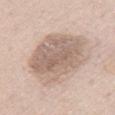follow-up=total-body-photography surveillance lesion; no biopsy
tile lighting=white-light
acquisition=15 mm crop, total-body photography
anatomic site=the chest
subject=male, aged approximately 60
lesion size=≈8.5 mm